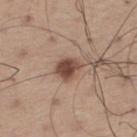Imaged during a routine full-body skin examination; the lesion was not biopsied and no histopathology is available.
About 3 mm across.
The lesion is on the right thigh.
The subject is a male aged 63–67.
Imaged with white-light lighting.
Automated tile analysis of the lesion measured a lesion color around L≈48 a*≈18 b*≈26 in CIELAB, a lesion–skin lightness drop of about 13, and a lesion-to-skin contrast of about 9.5 (normalized; higher = more distinct).
A region of skin cropped from a whole-body photographic capture, roughly 15 mm wide.